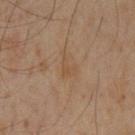<tbp_lesion>
<biopsy_status>not biopsied; imaged during a skin examination</biopsy_status>
<image>
  <source>total-body photography crop</source>
  <field_of_view_mm>15</field_of_view_mm>
</image>
<site>chest</site>
<automated_metrics>
  <cielab_L>45</cielab_L>
  <cielab_a>15</cielab_a>
  <cielab_b>29</cielab_b>
  <vs_skin_contrast_norm>4.5</vs_skin_contrast_norm>
  <border_irregularity_0_10>6.5</border_irregularity_0_10>
  <color_variation_0_10>1.0</color_variation_0_10>
</automated_metrics>
<lesion_size>
  <long_diameter_mm_approx>3.0</long_diameter_mm_approx>
</lesion_size>
<patient>
  <sex>male</sex>
  <age_approx>60</age_approx>
</patient>
</tbp_lesion>